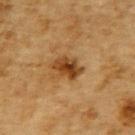Captured during whole-body skin photography for melanoma surveillance; the lesion was not biopsied.
The lesion's longest dimension is about 4 mm.
The tile uses cross-polarized illumination.
A male subject aged 83 or older.
A region of skin cropped from a whole-body photographic capture, roughly 15 mm wide.
The lesion is on the upper back.